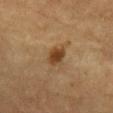Clinical impression: No biopsy was performed on this lesion — it was imaged during a full skin examination and was not determined to be concerning. Context: A male patient aged 83 or older. The lesion is located on the front of the torso. A region of skin cropped from a whole-body photographic capture, roughly 15 mm wide. The tile uses cross-polarized illumination.